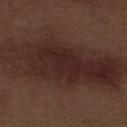Recorded during total-body skin imaging; not selected for excision or biopsy.
Imaged with white-light lighting.
Cropped from a total-body skin-imaging series; the visible field is about 15 mm.
The lesion is located on the left thigh.
A male patient aged 68–72.